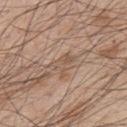The lesion's longest dimension is about 3 mm.
The lesion is on the back.
A roughly 15 mm field-of-view crop from a total-body skin photograph.
A male patient aged 43 to 47.
The tile uses white-light illumination.